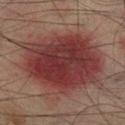notes = catalogued during a skin exam; not biopsied | automated metrics = an average lesion color of about L≈30 a*≈23 b*≈19 (CIELAB), about 11 CIELAB-L* units darker than the surrounding skin, and a normalized border contrast of about 10.5; a border-irregularity index near 2.5/10 and a within-lesion color-variation index near 5.5/10 | tile lighting = cross-polarized | imaging modality = total-body-photography crop, ~15 mm field of view | subject = male, in their mid-70s | anatomic site = the right lower leg | lesion size = about 10 mm.